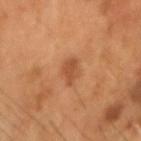{"biopsy_status": "not biopsied; imaged during a skin examination", "image": {"source": "total-body photography crop", "field_of_view_mm": 15}, "site": "head or neck", "patient": {"sex": "male", "age_approx": 55}}